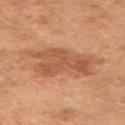Imaged during a routine full-body skin examination; the lesion was not biopsied and no histopathology is available. Imaged with cross-polarized lighting. Cropped from a whole-body photographic skin survey; the tile spans about 15 mm. From the left upper arm. An algorithmic analysis of the crop reported a within-lesion color-variation index near 2.5/10 and a peripheral color-asymmetry measure near 1. The software also gave a nevus-likeness score of about 15/100 and lesion-presence confidence of about 100/100. The patient is a female aged around 60.